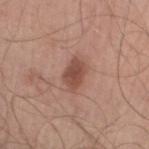| field | value |
|---|---|
| notes | imaged on a skin check; not biopsied |
| imaging modality | ~15 mm tile from a whole-body skin photo |
| diameter | about 3.5 mm |
| subject | male, approximately 50 years of age |
| anatomic site | the leg |
| image-analysis metrics | a lesion-to-skin contrast of about 8 (normalized; higher = more distinct); a border-irregularity rating of about 2.5/10, internal color variation of about 2 on a 0–10 scale, and peripheral color asymmetry of about 0.5; a nevus-likeness score of about 90/100 and a detector confidence of about 100 out of 100 that the crop contains a lesion |
| tile lighting | white-light illumination |